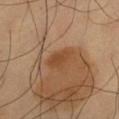<record>
  <biopsy_status>not biopsied; imaged during a skin examination</biopsy_status>
  <image>
    <source>total-body photography crop</source>
    <field_of_view_mm>15</field_of_view_mm>
  </image>
  <site>leg</site>
  <lesion_size>
    <long_diameter_mm_approx>3.0</long_diameter_mm_approx>
  </lesion_size>
  <patient>
    <sex>male</sex>
    <age_approx>60</age_approx>
  </patient>
  <automated_metrics>
    <cielab_L>44</cielab_L>
    <cielab_a>22</cielab_a>
    <cielab_b>35</cielab_b>
    <vs_skin_darker_L>7.0</vs_skin_darker_L>
    <vs_skin_contrast_norm>6.5</vs_skin_contrast_norm>
    <border_irregularity_0_10>3.5</border_irregularity_0_10>
    <color_variation_0_10>1.5</color_variation_0_10>
    <peripheral_color_asymmetry>0.5</peripheral_color_asymmetry>
    <nevus_likeness_0_100>90</nevus_likeness_0_100>
    <lesion_detection_confidence_0_100>100</lesion_detection_confidence_0_100>
  </automated_metrics>
</record>